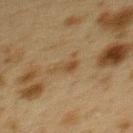The lesion was photographed on a routine skin check and not biopsied; there is no pathology result. Cropped from a whole-body photographic skin survey; the tile spans about 15 mm. The subject is a female aged approximately 40. The lesion is on the upper back.